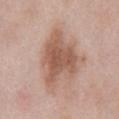Assessment:
The lesion was photographed on a routine skin check and not biopsied; there is no pathology result.
Image and clinical context:
The lesion's longest dimension is about 7 mm. A 15 mm crop from a total-body photograph taken for skin-cancer surveillance. Located on the abdomen. The lesion-visualizer software estimated an area of roughly 24 mm² and two-axis asymmetry of about 0.4. The analysis additionally found a border-irregularity index near 5/10 and internal color variation of about 3.5 on a 0–10 scale. The software also gave an automated nevus-likeness rating near 5 out of 100 and a lesion-detection confidence of about 100/100. Imaged with white-light lighting. A male subject, aged 53 to 57.